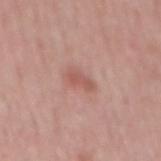<record>
<biopsy_status>not biopsied; imaged during a skin examination</biopsy_status>
<automated_metrics>
  <area_mm2_approx>3.5</area_mm2_approx>
  <eccentricity>0.85</eccentricity>
  <shape_asymmetry>0.3</shape_asymmetry>
  <cielab_L>55</cielab_L>
  <cielab_a>24</cielab_a>
  <cielab_b>25</cielab_b>
  <vs_skin_darker_L>8.0</vs_skin_darker_L>
  <vs_skin_contrast_norm>6.0</vs_skin_contrast_norm>
  <border_irregularity_0_10>3.0</border_irregularity_0_10>
  <color_variation_0_10>1.5</color_variation_0_10>
  <peripheral_color_asymmetry>0.5</peripheral_color_asymmetry>
</automated_metrics>
<lighting>white-light</lighting>
<patient>
  <sex>male</sex>
  <age_approx>50</age_approx>
</patient>
<site>mid back</site>
<image>
  <source>total-body photography crop</source>
  <field_of_view_mm>15</field_of_view_mm>
</image>
</record>